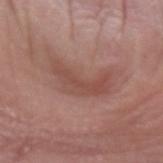Assessment: No biopsy was performed on this lesion — it was imaged during a full skin examination and was not determined to be concerning. Clinical summary: A 15 mm crop from a total-body photograph taken for skin-cancer surveillance. A male subject, aged 53 to 57. Automated tile analysis of the lesion measured a border-irregularity index near 7.5/10, a within-lesion color-variation index near 3/10, and peripheral color asymmetry of about 1.